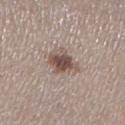This lesion was catalogued during total-body skin photography and was not selected for biopsy. The lesion-visualizer software estimated a footprint of about 8 mm², a shape eccentricity near 0.55, and two-axis asymmetry of about 0.25. It also reported a color-variation rating of about 5/10 and peripheral color asymmetry of about 1.5. A close-up tile cropped from a whole-body skin photograph, about 15 mm across. Longest diameter approximately 3.5 mm. The lesion is on the right lower leg. The tile uses white-light illumination. A female patient roughly 50 years of age.